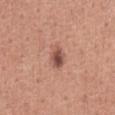Impression:
Imaged during a routine full-body skin examination; the lesion was not biopsied and no histopathology is available.
Context:
The lesion is located on the chest. Measured at roughly 3 mm in maximum diameter. A lesion tile, about 15 mm wide, cut from a 3D total-body photograph. The subject is a male aged around 45. The total-body-photography lesion software estimated a lesion color around L≈52 a*≈24 b*≈26 in CIELAB, about 12 CIELAB-L* units darker than the surrounding skin, and a normalized border contrast of about 8.5. And it measured a border-irregularity rating of about 2/10 and a color-variation rating of about 6/10. This is a white-light tile.